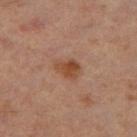Q: Was this lesion biopsied?
A: total-body-photography surveillance lesion; no biopsy
Q: What did automated image analysis measure?
A: border irregularity of about 3 on a 0–10 scale and internal color variation of about 3.5 on a 0–10 scale
Q: Lesion size?
A: ~3 mm (longest diameter)
Q: Where on the body is the lesion?
A: the left thigh
Q: Who is the patient?
A: male, aged 58 to 62
Q: What is the imaging modality?
A: total-body-photography crop, ~15 mm field of view
Q: Illumination type?
A: cross-polarized